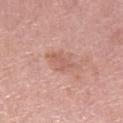The lesion was photographed on a routine skin check and not biopsied; there is no pathology result.